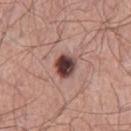Q: Was this lesion biopsied?
A: total-body-photography surveillance lesion; no biopsy
Q: What is the imaging modality?
A: ~15 mm tile from a whole-body skin photo
Q: What is the anatomic site?
A: the left thigh
Q: Patient demographics?
A: male, about 40 years old